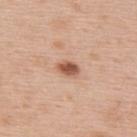Background: Imaged with white-light lighting. A male patient aged 58–62. Located on the upper back. Cropped from a whole-body photographic skin survey; the tile spans about 15 mm. The lesion's longest dimension is about 2.5 mm.